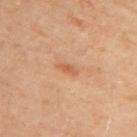Measured at roughly 2.5 mm in maximum diameter. The subject is a female aged approximately 50. The tile uses cross-polarized illumination. The lesion is located on the chest. An algorithmic analysis of the crop reported a border-irregularity index near 2.5/10, a within-lesion color-variation index near 1.5/10, and radial color variation of about 0.5. The software also gave a classifier nevus-likeness of about 5/100 and a detector confidence of about 100 out of 100 that the crop contains a lesion. Cropped from a total-body skin-imaging series; the visible field is about 15 mm.